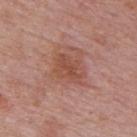Captured during whole-body skin photography for melanoma surveillance; the lesion was not biopsied. A close-up tile cropped from a whole-body skin photograph, about 15 mm across. Automated image analysis of the tile measured a border-irregularity rating of about 3.5/10, internal color variation of about 3 on a 0–10 scale, and radial color variation of about 1. The lesion is on the mid back. About 3.5 mm across. Imaged with white-light lighting. The patient is a male approximately 75 years of age.